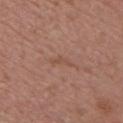Captured during whole-body skin photography for melanoma surveillance; the lesion was not biopsied.
The patient is a female aged 48 to 52.
The lesion is located on the chest.
A lesion tile, about 15 mm wide, cut from a 3D total-body photograph.